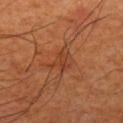The recorded lesion diameter is about 3.5 mm.
The lesion is on the left upper arm.
The total-body-photography lesion software estimated a lesion color around L≈42 a*≈26 b*≈36 in CIELAB and a normalized border contrast of about 5. It also reported border irregularity of about 4 on a 0–10 scale, a within-lesion color-variation index near 4/10, and peripheral color asymmetry of about 1.5. The analysis additionally found a lesion-detection confidence of about 95/100.
Imaged with cross-polarized lighting.
This image is a 15 mm lesion crop taken from a total-body photograph.
The subject is a male aged around 65.